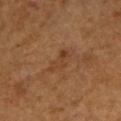No biopsy was performed on this lesion — it was imaged during a full skin examination and was not determined to be concerning.
A region of skin cropped from a whole-body photographic capture, roughly 15 mm wide.
The lesion is located on the left forearm.
The subject is a female aged 58 to 62.
Captured under cross-polarized illumination.
About 3.5 mm across.